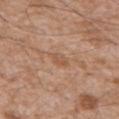Captured during whole-body skin photography for melanoma surveillance; the lesion was not biopsied.
Cropped from a whole-body photographic skin survey; the tile spans about 15 mm.
A male subject, aged 58–62.
The lesion-visualizer software estimated a border-irregularity index near 2/10, a color-variation rating of about 2.5/10, and peripheral color asymmetry of about 1. The analysis additionally found an automated nevus-likeness rating near 0 out of 100.
Located on the back.